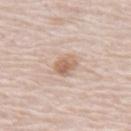  biopsy_status: not biopsied; imaged during a skin examination
  image:
    source: total-body photography crop
    field_of_view_mm: 15
  site: mid back
  patient:
    sex: male
    age_approx: 80